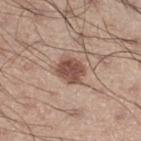This lesion was catalogued during total-body skin photography and was not selected for biopsy. The subject is a male aged 53 to 57. On the leg. Imaged with white-light lighting. Approximately 3.5 mm at its widest. Cropped from a total-body skin-imaging series; the visible field is about 15 mm.